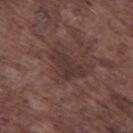Case summary:
• notes · no biopsy performed (imaged during a skin exam)
• subject · male, in their mid- to late 70s
• image · total-body-photography crop, ~15 mm field of view
• site · the left thigh